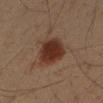Findings:
– image — ~15 mm tile from a whole-body skin photo
– patient — male, in their 50s
– automated lesion analysis — an area of roughly 13 mm² and a shape-asymmetry score of about 0.2 (0 = symmetric); an average lesion color of about L≈29 a*≈18 b*≈24 (CIELAB), roughly 11 lightness units darker than nearby skin, and a lesion-to-skin contrast of about 11 (normalized; higher = more distinct); a border-irregularity rating of about 2/10, a color-variation rating of about 3/10, and peripheral color asymmetry of about 0.5; a nevus-likeness score of about 100/100 and a detector confidence of about 100 out of 100 that the crop contains a lesion
– diameter — about 4 mm
– anatomic site — the right upper arm